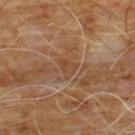Context:
Measured at roughly 2.5 mm in maximum diameter. Cropped from a total-body skin-imaging series; the visible field is about 15 mm. A male patient, aged around 60. Captured under cross-polarized illumination. The lesion is located on the front of the torso. Automated tile analysis of the lesion measured a lesion color around L≈43 a*≈19 b*≈30 in CIELAB, about 5 CIELAB-L* units darker than the surrounding skin, and a lesion-to-skin contrast of about 5 (normalized; higher = more distinct). And it measured border irregularity of about 3.5 on a 0–10 scale, internal color variation of about 2.5 on a 0–10 scale, and a peripheral color-asymmetry measure near 1. The software also gave an automated nevus-likeness rating near 0 out of 100 and a lesion-detection confidence of about 65/100.